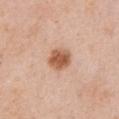Part of a total-body skin-imaging series; this lesion was reviewed on a skin check and was not flagged for biopsy. A 15 mm close-up tile from a total-body photography series done for melanoma screening. A female patient, in their 60s. From the chest.